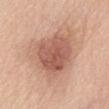Automated image analysis of the tile measured a lesion color around L≈57 a*≈24 b*≈28 in CIELAB and roughly 12 lightness units darker than nearby skin.
On the abdomen.
A female patient roughly 60 years of age.
Longest diameter approximately 6 mm.
The tile uses white-light illumination.
A 15 mm crop from a total-body photograph taken for skin-cancer surveillance.